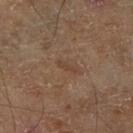follow-up: catalogued during a skin exam; not biopsied
lighting: cross-polarized
subject: male, aged approximately 70
acquisition: ~15 mm tile from a whole-body skin photo
lesion diameter: ≈2.5 mm
automated metrics: a footprint of about 2 mm² and an outline eccentricity of about 0.95 (0 = round, 1 = elongated); an average lesion color of about L≈40 a*≈17 b*≈27 (CIELAB), about 6 CIELAB-L* units darker than the surrounding skin, and a normalized border contrast of about 5
anatomic site: the leg